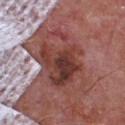Longest diameter approximately 8 mm.
Located on the chest.
A male patient, aged 63–67.
Cropped from a total-body skin-imaging series; the visible field is about 15 mm.
Captured under white-light illumination.